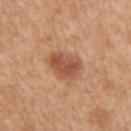Recorded during total-body skin imaging; not selected for excision or biopsy. The lesion is on the right upper arm. Longest diameter approximately 4 mm. A roughly 15 mm field-of-view crop from a total-body skin photograph. An algorithmic analysis of the crop reported a lesion area of about 9.5 mm², a shape eccentricity near 0.65, and a symmetry-axis asymmetry near 0.25. A female subject aged 53–57. Imaged with white-light lighting.